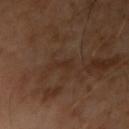Assessment: Captured during whole-body skin photography for melanoma surveillance; the lesion was not biopsied. Image and clinical context: The lesion-visualizer software estimated a footprint of about 3 mm² and an eccentricity of roughly 0.85. The recorded lesion diameter is about 2.5 mm. A lesion tile, about 15 mm wide, cut from a 3D total-body photograph. A male subject, aged 63–67.